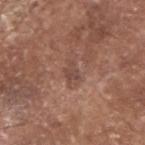image source = 15 mm crop, total-body photography | illumination = white-light | lesion diameter = ~3 mm (longest diameter) | location = the head or neck | patient = male, approximately 80 years of age.